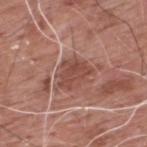Q: Was this lesion biopsied?
A: catalogued during a skin exam; not biopsied
Q: Illumination type?
A: white-light
Q: Automated lesion metrics?
A: a footprint of about 8.5 mm², an outline eccentricity of about 0.65 (0 = round, 1 = elongated), and a symmetry-axis asymmetry near 0.3
Q: What is the imaging modality?
A: 15 mm crop, total-body photography
Q: How large is the lesion?
A: ≈4 mm
Q: What are the patient's age and sex?
A: male, aged around 60
Q: Where on the body is the lesion?
A: the back